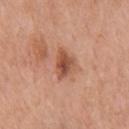| key | value |
|---|---|
| automated lesion analysis | a border-irregularity index near 3.5/10, internal color variation of about 5.5 on a 0–10 scale, and peripheral color asymmetry of about 1.5 |
| acquisition | total-body-photography crop, ~15 mm field of view |
| subject | male, about 70 years old |
| diameter | about 3.5 mm |
| site | the chest |